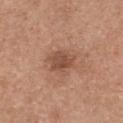workup: catalogued during a skin exam; not biopsied
imaging modality: ~15 mm tile from a whole-body skin photo
anatomic site: the chest
subject: female, aged approximately 30